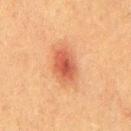Imaged during a routine full-body skin examination; the lesion was not biopsied and no histopathology is available. The tile uses cross-polarized illumination. On the chest. A male patient aged approximately 50. This image is a 15 mm lesion crop taken from a total-body photograph. Longest diameter approximately 5 mm.